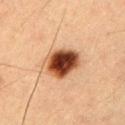Assessment: The lesion was tiled from a total-body skin photograph and was not biopsied. Context: The recorded lesion diameter is about 4.5 mm. The total-body-photography lesion software estimated a lesion color around L≈40 a*≈23 b*≈32 in CIELAB, a lesion–skin lightness drop of about 22, and a normalized border contrast of about 15.5. The lesion is located on the right upper arm. A roughly 15 mm field-of-view crop from a total-body skin photograph. The subject is a male in their mid-50s. The tile uses cross-polarized illumination.